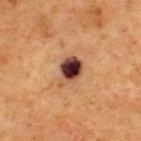| feature | finding |
|---|---|
| notes | no biopsy performed (imaged during a skin exam) |